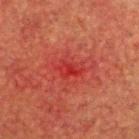workup = catalogued during a skin exam; not biopsied
image = ~15 mm tile from a whole-body skin photo
location = the head or neck
subject = male, approximately 60 years of age